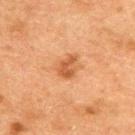Case summary:
• location — the upper back
• image source — total-body-photography crop, ~15 mm field of view
• lesion size — ≈3 mm
• subject — male, approximately 50 years of age
• automated lesion analysis — a mean CIELAB color near L≈45 a*≈22 b*≈34; an automated nevus-likeness rating near 55 out of 100
• illumination — cross-polarized illumination